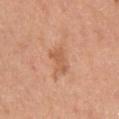Q: Is there a histopathology result?
A: imaged on a skin check; not biopsied
Q: What is the lesion's diameter?
A: ≈2.5 mm
Q: How was this image acquired?
A: total-body-photography crop, ~15 mm field of view
Q: What are the patient's age and sex?
A: male, aged 28–32
Q: How was the tile lit?
A: white-light illumination
Q: Lesion location?
A: the chest